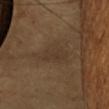follow-up: no biopsy performed (imaged during a skin exam) | location: the head or neck | acquisition: 15 mm crop, total-body photography | image-analysis metrics: a lesion color around L≈34 a*≈13 b*≈26 in CIELAB, about 5 CIELAB-L* units darker than the surrounding skin, and a lesion-to-skin contrast of about 4.5 (normalized; higher = more distinct); a border-irregularity index near 3.5/10, internal color variation of about 1.5 on a 0–10 scale, and radial color variation of about 0.5 | subject: female, aged 53–57.